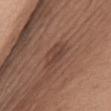No biopsy was performed on this lesion — it was imaged during a full skin examination and was not determined to be concerning. The lesion is located on the mid back. The total-body-photography lesion software estimated about 7 CIELAB-L* units darker than the surrounding skin and a lesion-to-skin contrast of about 6 (normalized; higher = more distinct). The software also gave a border-irregularity rating of about 2.5/10, internal color variation of about 3 on a 0–10 scale, and peripheral color asymmetry of about 1. A female subject approximately 65 years of age. A 15 mm crop from a total-body photograph taken for skin-cancer surveillance.